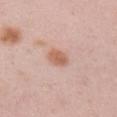follow-up: imaged on a skin check; not biopsied
subject: male, about 35 years old
body site: the right upper arm
acquisition: total-body-photography crop, ~15 mm field of view